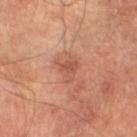Clinical impression:
Part of a total-body skin-imaging series; this lesion was reviewed on a skin check and was not flagged for biopsy.
Clinical summary:
This image is a 15 mm lesion crop taken from a total-body photograph. A male patient, aged approximately 65. On the left lower leg.